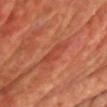This lesion was catalogued during total-body skin photography and was not selected for biopsy.
Located on the chest.
Imaged with cross-polarized lighting.
The recorded lesion diameter is about 4 mm.
A region of skin cropped from a whole-body photographic capture, roughly 15 mm wide.
The subject is a male aged approximately 60.